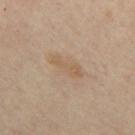Part of a total-body skin-imaging series; this lesion was reviewed on a skin check and was not flagged for biopsy. Cropped from a whole-body photographic skin survey; the tile spans about 15 mm. Located on the mid back. Captured under cross-polarized illumination. Automated image analysis of the tile measured radial color variation of about 0.5. The analysis additionally found a classifier nevus-likeness of about 0/100 and a lesion-detection confidence of about 100/100. A female patient in their mid- to late 40s. Approximately 4 mm at its widest.